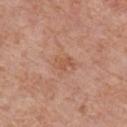Q: Was this lesion biopsied?
A: imaged on a skin check; not biopsied
Q: What is the lesion's diameter?
A: ≈2.5 mm
Q: Illumination type?
A: white-light illumination
Q: What is the anatomic site?
A: the chest
Q: What is the imaging modality?
A: 15 mm crop, total-body photography
Q: What are the patient's age and sex?
A: male, aged approximately 60